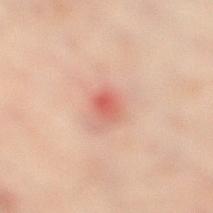Assessment:
Recorded during total-body skin imaging; not selected for excision or biopsy.
Background:
The lesion is on the left leg. This image is a 15 mm lesion crop taken from a total-body photograph. The subject is a male aged 48–52. An algorithmic analysis of the crop reported a lesion area of about 4 mm² and a shape eccentricity near 0.6. The analysis additionally found a border-irregularity index near 1.5/10, internal color variation of about 4 on a 0–10 scale, and radial color variation of about 1.5. This is a cross-polarized tile.